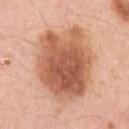The lesion's longest dimension is about 8 mm.
The lesion is located on the left upper arm.
A female patient aged 38 to 42.
A roughly 15 mm field-of-view crop from a total-body skin photograph.
Automated tile analysis of the lesion measured border irregularity of about 2 on a 0–10 scale, a color-variation rating of about 7/10, and a peripheral color-asymmetry measure near 2.5. The analysis additionally found a classifier nevus-likeness of about 90/100.